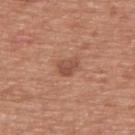Part of a total-body skin-imaging series; this lesion was reviewed on a skin check and was not flagged for biopsy.
The tile uses white-light illumination.
The patient is a male in their mid- to late 70s.
The recorded lesion diameter is about 2.5 mm.
A 15 mm close-up tile from a total-body photography series done for melanoma screening.
Located on the upper back.